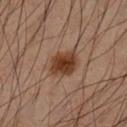  biopsy_status: not biopsied; imaged during a skin examination
  image:
    source: total-body photography crop
    field_of_view_mm: 15
  site: left lower leg
  patient:
    sex: male
    age_approx: 50
  lesion_size:
    long_diameter_mm_approx: 3.5